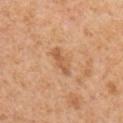Part of a total-body skin-imaging series; this lesion was reviewed on a skin check and was not flagged for biopsy. About 3.5 mm across. A male patient, in their mid- to late 50s. The lesion is on the chest. A 15 mm close-up extracted from a 3D total-body photography capture. The tile uses white-light illumination.